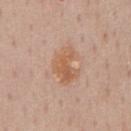Assessment: Imaged during a routine full-body skin examination; the lesion was not biopsied and no histopathology is available. Clinical summary: The lesion is on the front of the torso. A 15 mm crop from a total-body photograph taken for skin-cancer surveillance. A male patient, roughly 60 years of age. About 4.5 mm across. Automated tile analysis of the lesion measured an average lesion color of about L≈59 a*≈21 b*≈33 (CIELAB), a lesion–skin lightness drop of about 8, and a normalized lesion–skin contrast near 7. The software also gave a border-irregularity index near 5/10, internal color variation of about 4 on a 0–10 scale, and a peripheral color-asymmetry measure near 1.5. This is a white-light tile.